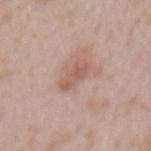This lesion was catalogued during total-body skin photography and was not selected for biopsy.
A female subject in their 30s.
On the mid back.
Captured under white-light illumination.
The lesion's longest dimension is about 4 mm.
A 15 mm close-up extracted from a 3D total-body photography capture.